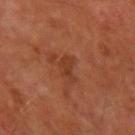The lesion was tiled from a total-body skin photograph and was not biopsied.
Cropped from a whole-body photographic skin survey; the tile spans about 15 mm.
Approximately 3 mm at its widest.
A male patient aged around 50.
On the left upper arm.
Captured under cross-polarized illumination.